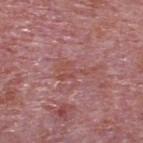Impression: This lesion was catalogued during total-body skin photography and was not selected for biopsy. Image and clinical context: The lesion is on the upper back. Cropped from a whole-body photographic skin survey; the tile spans about 15 mm. About 4 mm across. A male patient approximately 75 years of age. The tile uses white-light illumination. Automated image analysis of the tile measured a footprint of about 5 mm² and a shape-asymmetry score of about 0.65 (0 = symmetric). The analysis additionally found a mean CIELAB color near L≈49 a*≈26 b*≈24, roughly 5 lightness units darker than nearby skin, and a normalized border contrast of about 5. And it measured an automated nevus-likeness rating near 0 out of 100 and a detector confidence of about 60 out of 100 that the crop contains a lesion.